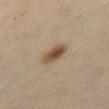Impression:
Imaged during a routine full-body skin examination; the lesion was not biopsied and no histopathology is available.
Context:
Measured at roughly 3.5 mm in maximum diameter. The subject is a male in their 50s. Imaged with cross-polarized lighting. A 15 mm close-up extracted from a 3D total-body photography capture. An algorithmic analysis of the crop reported two-axis asymmetry of about 0.2. And it measured an average lesion color of about L≈49 a*≈16 b*≈31 (CIELAB), a lesion–skin lightness drop of about 12, and a normalized lesion–skin contrast near 9.5. The software also gave a nevus-likeness score of about 100/100. The lesion is located on the abdomen.